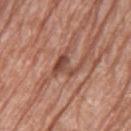notes: catalogued during a skin exam; not biopsied
tile lighting: white-light
patient: female, in their mid- to late 70s
TBP lesion metrics: a footprint of about 5.5 mm² and a shape-asymmetry score of about 0.7 (0 = symmetric); an average lesion color of about L≈47 a*≈24 b*≈28 (CIELAB), a lesion–skin lightness drop of about 10, and a lesion-to-skin contrast of about 7.5 (normalized; higher = more distinct); an automated nevus-likeness rating near 0 out of 100
acquisition: ~15 mm tile from a whole-body skin photo
site: the left thigh